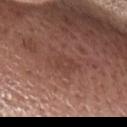A male patient, aged around 65.
The lesion is on the head or neck.
A close-up tile cropped from a whole-body skin photograph, about 15 mm across.
Automated image analysis of the tile measured an area of roughly 7 mm², a shape eccentricity near 0.85, and a shape-asymmetry score of about 0.3 (0 = symmetric).
Imaged with white-light lighting.